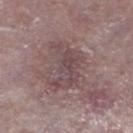The lesion was photographed on a routine skin check and not biopsied; there is no pathology result. Automated tile analysis of the lesion measured an average lesion color of about L≈46 a*≈17 b*≈15 (CIELAB), about 8 CIELAB-L* units darker than the surrounding skin, and a normalized border contrast of about 6. The analysis additionally found a nevus-likeness score of about 0/100. A male subject aged around 75. Located on the left lower leg. The tile uses white-light illumination. This image is a 15 mm lesion crop taken from a total-body photograph.